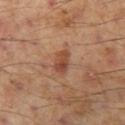The lesion was photographed on a routine skin check and not biopsied; there is no pathology result.
Longest diameter approximately 3 mm.
An algorithmic analysis of the crop reported a border-irregularity rating of about 2/10, a within-lesion color-variation index near 5/10, and a peripheral color-asymmetry measure near 1.5. The software also gave an automated nevus-likeness rating near 45 out of 100.
A 15 mm close-up tile from a total-body photography series done for melanoma screening.
Captured under cross-polarized illumination.
The subject is a male aged around 55.
On the left thigh.